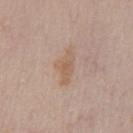biopsy_status: not biopsied; imaged during a skin examination
patient:
  sex: male
  age_approx: 60
automated_metrics:
  area_mm2_approx: 6.5
  eccentricity: 0.9
  shape_asymmetry: 0.4
  border_irregularity_0_10: 4.5
  color_variation_0_10: 2.0
  peripheral_color_asymmetry: 0.5
lesion_size:
  long_diameter_mm_approx: 4.5
image:
  source: total-body photography crop
  field_of_view_mm: 15
site: front of the torso